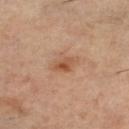• biopsy status · imaged on a skin check; not biopsied
• image-analysis metrics · a lesion color around L≈54 a*≈23 b*≈35 in CIELAB and a lesion–skin lightness drop of about 10; border irregularity of about 2 on a 0–10 scale, a within-lesion color-variation index near 5/10, and a peripheral color-asymmetry measure near 1.5; an automated nevus-likeness rating near 60 out of 100 and lesion-presence confidence of about 100/100
• acquisition · 15 mm crop, total-body photography
• location · the left lower leg
• subject · female, approximately 60 years of age
• illumination · cross-polarized
• diameter · about 3 mm